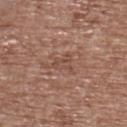Clinical impression:
The lesion was tiled from a total-body skin photograph and was not biopsied.
Context:
The patient is a female aged approximately 75. Approximately 2.5 mm at its widest. A lesion tile, about 15 mm wide, cut from a 3D total-body photograph. Automated image analysis of the tile measured an average lesion color of about L≈48 a*≈21 b*≈27 (CIELAB). The software also gave a border-irregularity index near 4/10, internal color variation of about 2 on a 0–10 scale, and peripheral color asymmetry of about 0.5. From the upper back. Captured under white-light illumination.